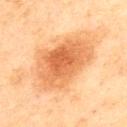Recorded during total-body skin imaging; not selected for excision or biopsy. Imaged with cross-polarized lighting. A male subject, aged 43 to 47. From the upper back. A roughly 15 mm field-of-view crop from a total-body skin photograph.